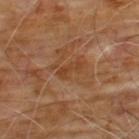The lesion was tiled from a total-body skin photograph and was not biopsied. Cropped from a total-body skin-imaging series; the visible field is about 15 mm. On the front of the torso. A male subject, aged approximately 60. The lesion-visualizer software estimated an average lesion color of about L≈40 a*≈21 b*≈33 (CIELAB) and about 6 CIELAB-L* units darker than the surrounding skin. The software also gave a border-irregularity index near 4/10 and a within-lesion color-variation index near 1/10. The recorded lesion diameter is about 3 mm. This is a cross-polarized tile.